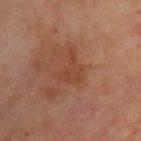The lesion was photographed on a routine skin check and not biopsied; there is no pathology result.
Located on the upper back.
About 4 mm across.
A roughly 15 mm field-of-view crop from a total-body skin photograph.
Captured under cross-polarized illumination.
A male subject, roughly 70 years of age.